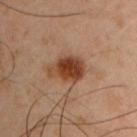Q: Was a biopsy performed?
A: no biopsy performed (imaged during a skin exam)
Q: What is the anatomic site?
A: the left upper arm
Q: Who is the patient?
A: male, aged approximately 45
Q: Illumination type?
A: cross-polarized illumination
Q: What kind of image is this?
A: ~15 mm crop, total-body skin-cancer survey
Q: How large is the lesion?
A: ~4.5 mm (longest diameter)